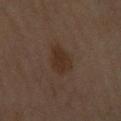Q: Was this lesion biopsied?
A: total-body-photography surveillance lesion; no biopsy
Q: Lesion location?
A: the left forearm
Q: What is the lesion's diameter?
A: ≈3.5 mm
Q: Automated lesion metrics?
A: a footprint of about 7.5 mm², an outline eccentricity of about 0.7 (0 = round, 1 = elongated), and a shape-asymmetry score of about 0.25 (0 = symmetric); a lesion color around L≈25 a*≈13 b*≈21 in CIELAB and roughly 6 lightness units darker than nearby skin; border irregularity of about 2.5 on a 0–10 scale, a within-lesion color-variation index near 2/10, and radial color variation of about 0.5
Q: What kind of image is this?
A: 15 mm crop, total-body photography
Q: Who is the patient?
A: female, approximately 60 years of age